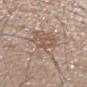Captured during whole-body skin photography for melanoma surveillance; the lesion was not biopsied. From the mid back. A 15 mm crop from a total-body photograph taken for skin-cancer surveillance. The lesion's longest dimension is about 7.5 mm. A male subject aged 53 to 57. Captured under white-light illumination.